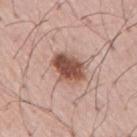Captured during whole-body skin photography for melanoma surveillance; the lesion was not biopsied. This is a white-light tile. A roughly 15 mm field-of-view crop from a total-body skin photograph. The patient is a male in their mid- to late 50s. Measured at roughly 4.5 mm in maximum diameter. Automated tile analysis of the lesion measured a footprint of about 10 mm², a shape eccentricity near 0.7, and a symmetry-axis asymmetry near 0.2. It also reported a mean CIELAB color near L≈51 a*≈22 b*≈27, about 16 CIELAB-L* units darker than the surrounding skin, and a normalized lesion–skin contrast near 10.5. It also reported internal color variation of about 5 on a 0–10 scale.